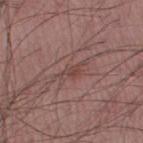Q: Was a biopsy performed?
A: total-body-photography surveillance lesion; no biopsy
Q: What lighting was used for the tile?
A: white-light
Q: How was this image acquired?
A: ~15 mm crop, total-body skin-cancer survey
Q: How large is the lesion?
A: about 2.5 mm
Q: Who is the patient?
A: male, roughly 50 years of age
Q: Lesion location?
A: the right thigh